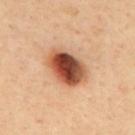Clinical impression: Recorded during total-body skin imaging; not selected for excision or biopsy. Context: A male patient in their 40s. Located on the upper back. Cropped from a whole-body photographic skin survey; the tile spans about 15 mm. Measured at roughly 5 mm in maximum diameter. Imaged with cross-polarized lighting.